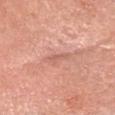The lesion was tiled from a total-body skin photograph and was not biopsied. From the head or neck. Automated image analysis of the tile measured a lesion area of about 2 mm² and an outline eccentricity of about 0.95 (0 = round, 1 = elongated). The analysis additionally found a border-irregularity index near 4.5/10, a color-variation rating of about 0/10, and peripheral color asymmetry of about 0. And it measured a classifier nevus-likeness of about 0/100. A 15 mm crop from a total-body photograph taken for skin-cancer surveillance. A female patient, in their mid- to late 70s.